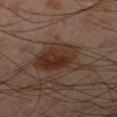Clinical impression: Part of a total-body skin-imaging series; this lesion was reviewed on a skin check and was not flagged for biopsy. Context: Longest diameter approximately 7 mm. A 15 mm crop from a total-body photograph taken for skin-cancer surveillance. A male subject aged around 55. Imaged with cross-polarized lighting. From the right lower leg.